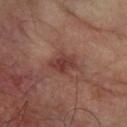Assessment:
Recorded during total-body skin imaging; not selected for excision or biopsy.
Context:
From the right forearm. A 15 mm close-up extracted from a 3D total-body photography capture. A male subject approximately 65 years of age.